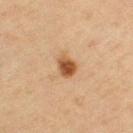A 15 mm close-up tile from a total-body photography series done for melanoma screening.
Approximately 2.5 mm at its widest.
This is a cross-polarized tile.
A female patient, aged around 45.
From the left upper arm.